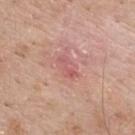This lesion was catalogued during total-body skin photography and was not selected for biopsy. The tile uses white-light illumination. On the upper back. An algorithmic analysis of the crop reported a footprint of about 5 mm² and a symmetry-axis asymmetry near 0.3. The software also gave a peripheral color-asymmetry measure near 1. A male subject aged 58 to 62. Measured at roughly 3.5 mm in maximum diameter. A lesion tile, about 15 mm wide, cut from a 3D total-body photograph.